Automated tile analysis of the lesion measured an average lesion color of about L≈21 a*≈21 b*≈22 (CIELAB) and a lesion–skin lightness drop of about 17. The analysis additionally found lesion-presence confidence of about 100/100 · a male subject aged around 50 · located on the back · a region of skin cropped from a whole-body photographic capture, roughly 15 mm wide · imaged with cross-polarized lighting · the recorded lesion diameter is about 6.5 mm: 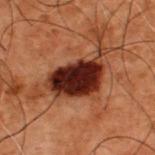histopathologic diagnosis=a dysplastic (Clark) nevus.